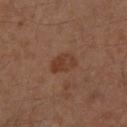No biopsy was performed on this lesion — it was imaged during a full skin examination and was not determined to be concerning.
A 15 mm crop from a total-body photograph taken for skin-cancer surveillance.
From the leg.
A male subject, about 60 years old.